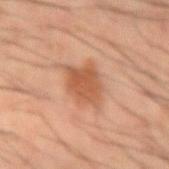Impression:
The lesion was tiled from a total-body skin photograph and was not biopsied.
Background:
A roughly 15 mm field-of-view crop from a total-body skin photograph. The subject is a male roughly 60 years of age. Located on the arm.